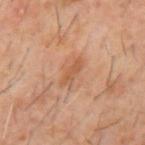{
  "biopsy_status": "not biopsied; imaged during a skin examination",
  "site": "mid back",
  "image": {
    "source": "total-body photography crop",
    "field_of_view_mm": 15
  },
  "patient": {
    "sex": "male",
    "age_approx": 60
  },
  "lighting": "cross-polarized"
}